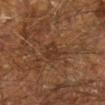image:
  source: total-body photography crop
  field_of_view_mm: 15
site: left lower leg
lighting: cross-polarized
patient:
  sex: male
  age_approx: 60
lesion_size:
  long_diameter_mm_approx: 2.5